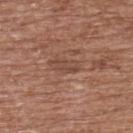biopsy status: total-body-photography surveillance lesion; no biopsy
patient: male, aged 73–77
imaging modality: ~15 mm tile from a whole-body skin photo
image-analysis metrics: a footprint of about 3.5 mm², an eccentricity of roughly 0.85, and two-axis asymmetry of about 0.25; a lesion color around L≈46 a*≈20 b*≈27 in CIELAB and about 7 CIELAB-L* units darker than the surrounding skin; a border-irregularity index near 2.5/10, a within-lesion color-variation index near 2.5/10, and a peripheral color-asymmetry measure near 1; a nevus-likeness score of about 5/100
location: the upper back
illumination: white-light illumination